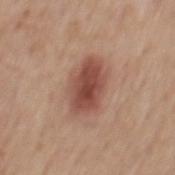Q: Is there a histopathology result?
A: imaged on a skin check; not biopsied
Q: What is the lesion's diameter?
A: about 5.5 mm
Q: What are the patient's age and sex?
A: male, in their mid-60s
Q: What is the imaging modality?
A: ~15 mm crop, total-body skin-cancer survey
Q: Automated lesion metrics?
A: a lesion area of about 14 mm², an outline eccentricity of about 0.8 (0 = round, 1 = elongated), and a shape-asymmetry score of about 0.2 (0 = symmetric); a border-irregularity index near 2.5/10 and a peripheral color-asymmetry measure near 1.5; an automated nevus-likeness rating near 100 out of 100
Q: What is the anatomic site?
A: the mid back
Q: How was the tile lit?
A: white-light